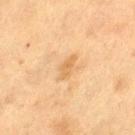The lesion was photographed on a routine skin check and not biopsied; there is no pathology result. A female subject, about 40 years old. Imaged with cross-polarized lighting. The lesion's longest dimension is about 3 mm. The lesion is located on the left thigh. A lesion tile, about 15 mm wide, cut from a 3D total-body photograph.